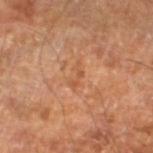The lesion was tiled from a total-body skin photograph and was not biopsied. A region of skin cropped from a whole-body photographic capture, roughly 15 mm wide. The lesion's longest dimension is about 3 mm. A male subject, approximately 60 years of age. Captured under cross-polarized illumination. Automated image analysis of the tile measured a footprint of about 3 mm² and two-axis asymmetry of about 0.5. The software also gave a border-irregularity index near 6.5/10 and a color-variation rating of about 0/10. Located on the right leg.